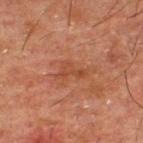notes: total-body-photography surveillance lesion; no biopsy | image source: ~15 mm crop, total-body skin-cancer survey | tile lighting: cross-polarized | patient: male, about 50 years old | site: the upper back | automated lesion analysis: a lesion area of about 4.5 mm², a shape eccentricity near 0.85, and a symmetry-axis asymmetry near 0.65; a border-irregularity index near 7/10, a color-variation rating of about 1.5/10, and peripheral color asymmetry of about 0.5; a lesion-detection confidence of about 100/100.